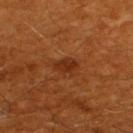  biopsy_status: not biopsied; imaged during a skin examination
  lighting: cross-polarized
  image:
    source: total-body photography crop
    field_of_view_mm: 15
  patient:
    sex: male
    age_approx: 60
  site: upper back